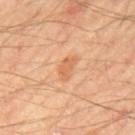Captured during whole-body skin photography for melanoma surveillance; the lesion was not biopsied.
The lesion is on the mid back.
A male patient, aged around 65.
A 15 mm close-up extracted from a 3D total-body photography capture.